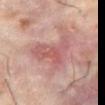Assessment:
The lesion was tiled from a total-body skin photograph and was not biopsied.
Image and clinical context:
Imaged with cross-polarized lighting. A male subject, about 60 years old. A region of skin cropped from a whole-body photographic capture, roughly 15 mm wide. The recorded lesion diameter is about 5.5 mm. The lesion-visualizer software estimated a footprint of about 13 mm², an eccentricity of roughly 0.7, and two-axis asymmetry of about 0.6. It also reported a lesion color around L≈52 a*≈24 b*≈21 in CIELAB, roughly 8 lightness units darker than nearby skin, and a lesion-to-skin contrast of about 6 (normalized; higher = more distinct). The analysis additionally found a border-irregularity index near 7/10, a within-lesion color-variation index near 5.5/10, and radial color variation of about 2. From the abdomen.